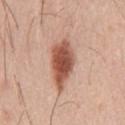| feature | finding |
|---|---|
| automated metrics | a nevus-likeness score of about 100/100 and lesion-presence confidence of about 100/100 |
| patient | male, approximately 45 years of age |
| anatomic site | the chest |
| acquisition | ~15 mm crop, total-body skin-cancer survey |
| lighting | white-light illumination |
| lesion size | ~6 mm (longest diameter) |